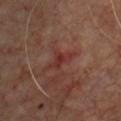notes: catalogued during a skin exam; not biopsied
automated metrics: an eccentricity of roughly 0.85 and two-axis asymmetry of about 0.6; a mean CIELAB color near L≈32 a*≈26 b*≈23, roughly 6 lightness units darker than nearby skin, and a lesion-to-skin contrast of about 6 (normalized; higher = more distinct)
anatomic site: the chest
subject: male, aged 68 to 72
size: about 3.5 mm
acquisition: ~15 mm tile from a whole-body skin photo